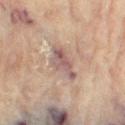notes: catalogued during a skin exam; not biopsied | image: total-body-photography crop, ~15 mm field of view | automated metrics: a footprint of about 7 mm², an outline eccentricity of about 0.85 (0 = round, 1 = elongated), and a shape-asymmetry score of about 0.4 (0 = symmetric); border irregularity of about 4.5 on a 0–10 scale and internal color variation of about 5.5 on a 0–10 scale | patient: female, about 80 years old | location: the right thigh.